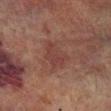  biopsy_status: not biopsied; imaged during a skin examination
  site: right lower leg
  lesion_size:
    long_diameter_mm_approx: 4.5
  image:
    source: total-body photography crop
    field_of_view_mm: 15
  patient:
    sex: male
    age_approx: 75
  lighting: cross-polarized